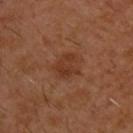Located on the upper back. Cropped from a whole-body photographic skin survey; the tile spans about 15 mm. The recorded lesion diameter is about 3.5 mm. A male subject, roughly 30 years of age. Automated image analysis of the tile measured a footprint of about 7 mm², an eccentricity of roughly 0.65, and a symmetry-axis asymmetry near 0.25. The software also gave about 7 CIELAB-L* units darker than the surrounding skin. The software also gave a classifier nevus-likeness of about 0/100 and a detector confidence of about 100 out of 100 that the crop contains a lesion.